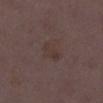Part of a total-body skin-imaging series; this lesion was reviewed on a skin check and was not flagged for biopsy. Longest diameter approximately 3.5 mm. A roughly 15 mm field-of-view crop from a total-body skin photograph. The lesion is on the right lower leg. The patient is a female aged around 30.